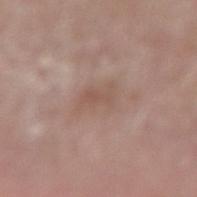Notes:
* notes · no biopsy performed (imaged during a skin exam)
* anatomic site · the right lower leg
* tile lighting · white-light illumination
* TBP lesion metrics · a footprint of about 7.5 mm² and two-axis asymmetry of about 0.35; border irregularity of about 4.5 on a 0–10 scale, a within-lesion color-variation index near 2/10, and radial color variation of about 0.5; a classifier nevus-likeness of about 0/100 and a lesion-detection confidence of about 100/100
* image source · ~15 mm crop, total-body skin-cancer survey
* lesion size · about 4.5 mm
* subject · male, aged approximately 65